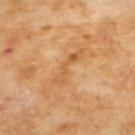No biopsy was performed on this lesion — it was imaged during a full skin examination and was not determined to be concerning. Automated tile analysis of the lesion measured an eccentricity of roughly 0.95 and a shape-asymmetry score of about 0.55 (0 = symmetric). And it measured an average lesion color of about L≈58 a*≈23 b*≈42 (CIELAB), roughly 7 lightness units darker than nearby skin, and a normalized lesion–skin contrast near 5. It also reported a border-irregularity index near 7.5/10 and a peripheral color-asymmetry measure near 0.5. The analysis additionally found a classifier nevus-likeness of about 0/100. A male patient, aged 68–72. Located on the upper back. Captured under cross-polarized illumination. The recorded lesion diameter is about 5 mm. A region of skin cropped from a whole-body photographic capture, roughly 15 mm wide.